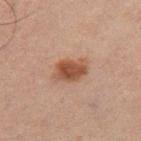Assessment: Part of a total-body skin-imaging series; this lesion was reviewed on a skin check and was not flagged for biopsy.